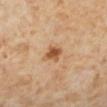Impression: No biopsy was performed on this lesion — it was imaged during a full skin examination and was not determined to be concerning. Acquisition and patient details: Imaged with cross-polarized lighting. A roughly 15 mm field-of-view crop from a total-body skin photograph. A female patient, in their 50s. The recorded lesion diameter is about 2.5 mm. On the left lower leg. Automated tile analysis of the lesion measured a lesion area of about 5 mm² and an eccentricity of roughly 0.35. And it measured an average lesion color of about L≈56 a*≈23 b*≈38 (CIELAB), about 12 CIELAB-L* units darker than the surrounding skin, and a normalized border contrast of about 8.5. And it measured internal color variation of about 3.5 on a 0–10 scale and peripheral color asymmetry of about 1.5. The analysis additionally found an automated nevus-likeness rating near 95 out of 100 and a lesion-detection confidence of about 100/100.